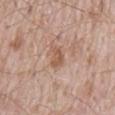• tile lighting — white-light illumination
• image-analysis metrics — a lesion area of about 4 mm², an eccentricity of roughly 0.85, and a shape-asymmetry score of about 0.35 (0 = symmetric); an automated nevus-likeness rating near 0 out of 100 and a lesion-detection confidence of about 100/100
• lesion size — about 3 mm
• patient — male, in their mid-60s
• site — the back
• image — 15 mm crop, total-body photography